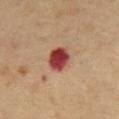Clinical summary: This is a cross-polarized tile. A male patient, in their mid-60s. Automated image analysis of the tile measured a lesion color around L≈42 a*≈32 b*≈27 in CIELAB and a normalized border contrast of about 13.5. And it measured a border-irregularity index near 1.5/10, a within-lesion color-variation index near 5/10, and peripheral color asymmetry of about 1.5. The software also gave an automated nevus-likeness rating near 0 out of 100 and a detector confidence of about 100 out of 100 that the crop contains a lesion. A region of skin cropped from a whole-body photographic capture, roughly 15 mm wide. Measured at roughly 3.5 mm in maximum diameter.